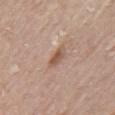The lesion was tiled from a total-body skin photograph and was not biopsied. The patient is a female in their 60s. The lesion's longest dimension is about 2.5 mm. A lesion tile, about 15 mm wide, cut from a 3D total-body photograph. Automated tile analysis of the lesion measured border irregularity of about 2 on a 0–10 scale, a within-lesion color-variation index near 4/10, and a peripheral color-asymmetry measure near 1.5. And it measured a nevus-likeness score of about 45/100. On the left upper arm.